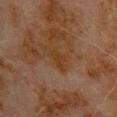biopsy status — imaged on a skin check; not biopsied | subject — male, aged 78 to 82 | lighting — cross-polarized | diameter — ~2.5 mm (longest diameter) | anatomic site — the upper back | image — ~15 mm tile from a whole-body skin photo.